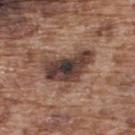{"biopsy_status": "not biopsied; imaged during a skin examination", "image": {"source": "total-body photography crop", "field_of_view_mm": 15}, "automated_metrics": {"vs_skin_contrast_norm": 12.0, "border_irregularity_0_10": 5.5, "color_variation_0_10": 8.0, "peripheral_color_asymmetry": 2.0}, "site": "upper back", "lighting": "white-light", "patient": {"sex": "male", "age_approx": 75}}